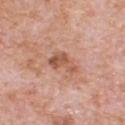follow-up: imaged on a skin check; not biopsied
image-analysis metrics: a lesion color around L≈55 a*≈23 b*≈31 in CIELAB and a normalized lesion–skin contrast near 7.5; a classifier nevus-likeness of about 0/100 and lesion-presence confidence of about 100/100
diameter: ≈4 mm
illumination: white-light
image: ~15 mm crop, total-body skin-cancer survey
subject: male, in their 80s
anatomic site: the upper back